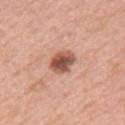Clinical impression:
Recorded during total-body skin imaging; not selected for excision or biopsy.
Image and clinical context:
The lesion is on the chest. Approximately 3 mm at its widest. A male subject aged approximately 60. Captured under white-light illumination. A 15 mm close-up tile from a total-body photography series done for melanoma screening.